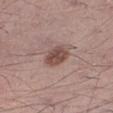Findings:
– illumination · white-light
– size · ≈3 mm
– image source · 15 mm crop, total-body photography
– subject · male, aged 58 to 62
– location · the leg